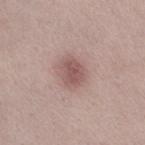Acquisition and patient details: The lesion is on the right thigh. A female subject, aged around 40. An algorithmic analysis of the crop reported a mean CIELAB color near L≈54 a*≈20 b*≈21, a lesion–skin lightness drop of about 10, and a lesion-to-skin contrast of about 7 (normalized; higher = more distinct). And it measured an automated nevus-likeness rating near 85 out of 100 and a detector confidence of about 100 out of 100 that the crop contains a lesion. A lesion tile, about 15 mm wide, cut from a 3D total-body photograph.